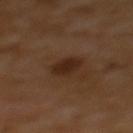Part of a total-body skin-imaging series; this lesion was reviewed on a skin check and was not flagged for biopsy.
The lesion-visualizer software estimated an area of roughly 6.5 mm², an eccentricity of roughly 0.2, and two-axis asymmetry of about 0.15. The software also gave a border-irregularity rating of about 1/10, a within-lesion color-variation index near 2/10, and radial color variation of about 0.5. And it measured a classifier nevus-likeness of about 95/100 and lesion-presence confidence of about 100/100.
The lesion is on the upper back.
Approximately 3 mm at its widest.
The patient is a female roughly 55 years of age.
A lesion tile, about 15 mm wide, cut from a 3D total-body photograph.
Imaged with cross-polarized lighting.